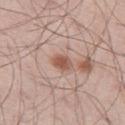The lesion was photographed on a routine skin check and not biopsied; there is no pathology result.
This is a white-light tile.
This image is a 15 mm lesion crop taken from a total-body photograph.
A male patient, in their 60s.
The total-body-photography lesion software estimated a lesion area of about 4 mm² and a shape-asymmetry score of about 0.15 (0 = symmetric). It also reported a lesion color around L≈55 a*≈21 b*≈27 in CIELAB, about 11 CIELAB-L* units darker than the surrounding skin, and a normalized lesion–skin contrast near 8. It also reported a border-irregularity index near 1.5/10, a color-variation rating of about 3.5/10, and radial color variation of about 1. The software also gave a nevus-likeness score of about 95/100 and a detector confidence of about 100 out of 100 that the crop contains a lesion.
The lesion is on the left thigh.